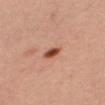This lesion was catalogued during total-body skin photography and was not selected for biopsy.
Cropped from a whole-body photographic skin survey; the tile spans about 15 mm.
A female subject aged 43 to 47.
Automated tile analysis of the lesion measured a border-irregularity index near 2/10, a within-lesion color-variation index near 3.5/10, and radial color variation of about 1. The analysis additionally found a classifier nevus-likeness of about 100/100 and a detector confidence of about 100 out of 100 that the crop contains a lesion.
On the right thigh.